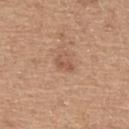Q: Was a biopsy performed?
A: total-body-photography surveillance lesion; no biopsy
Q: Lesion size?
A: about 2.5 mm
Q: How was the tile lit?
A: white-light
Q: Patient demographics?
A: male, aged around 65
Q: What kind of image is this?
A: 15 mm crop, total-body photography
Q: Lesion location?
A: the upper back